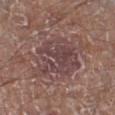Assessment: The lesion was photographed on a routine skin check and not biopsied; there is no pathology result. Acquisition and patient details: About 6 mm across. A close-up tile cropped from a whole-body skin photograph, about 15 mm across. This is a white-light tile. The total-body-photography lesion software estimated a lesion area of about 22 mm², an outline eccentricity of about 0.55 (0 = round, 1 = elongated), and two-axis asymmetry of about 0.2. It also reported a lesion color around L≈43 a*≈18 b*≈17 in CIELAB, a lesion–skin lightness drop of about 8, and a lesion-to-skin contrast of about 7 (normalized; higher = more distinct). And it measured a border-irregularity index near 3/10, a within-lesion color-variation index near 4.5/10, and radial color variation of about 1.5. It also reported a lesion-detection confidence of about 85/100. The lesion is on the left lower leg. The patient is a male aged approximately 80.